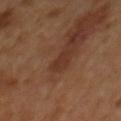notes = imaged on a skin check; not biopsied | image = ~15 mm crop, total-body skin-cancer survey | image-analysis metrics = an eccentricity of roughly 0.85; a border-irregularity rating of about 3.5/10 and a within-lesion color-variation index near 0/10; lesion-presence confidence of about 95/100 | body site = the mid back | size = ≈2.5 mm | illumination = cross-polarized illumination | subject = male, about 65 years old.